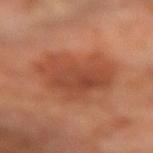Q: What kind of image is this?
A: ~15 mm tile from a whole-body skin photo
Q: What are the patient's age and sex?
A: male, approximately 70 years of age
Q: What did automated image analysis measure?
A: a lesion area of about 26 mm², an eccentricity of roughly 0.45, and two-axis asymmetry of about 0.35; a mean CIELAB color near L≈46 a*≈27 b*≈33 and a lesion–skin lightness drop of about 9; an automated nevus-likeness rating near 90 out of 100
Q: What is the anatomic site?
A: the left forearm
Q: Illumination type?
A: cross-polarized